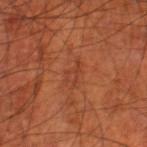The patient is a male aged 68–72. A 15 mm close-up tile from a total-body photography series done for melanoma screening. Automated tile analysis of the lesion measured an automated nevus-likeness rating near 0 out of 100 and a lesion-detection confidence of about 100/100. Located on the right thigh. Approximately 3 mm at its widest. Captured under cross-polarized illumination.